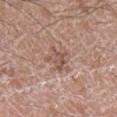Assessment: Recorded during total-body skin imaging; not selected for excision or biopsy. Image and clinical context: The patient is a male about 60 years old. Cropped from a total-body skin-imaging series; the visible field is about 15 mm. The lesion is located on the right lower leg.